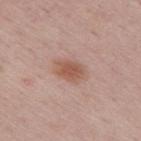Imaged during a routine full-body skin examination; the lesion was not biopsied and no histopathology is available.
The lesion is on the upper back.
A lesion tile, about 15 mm wide, cut from a 3D total-body photograph.
The patient is a female aged 48–52.